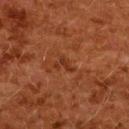notes: imaged on a skin check; not biopsied | anatomic site: the upper back | image source: ~15 mm tile from a whole-body skin photo | subject: female, in their 50s.